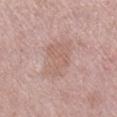Case summary:
- biopsy status: no biopsy performed (imaged during a skin exam)
- size: about 5 mm
- image: ~15 mm tile from a whole-body skin photo
- TBP lesion metrics: border irregularity of about 5 on a 0–10 scale, a color-variation rating of about 2/10, and peripheral color asymmetry of about 0.5; a lesion-detection confidence of about 100/100
- subject: female, aged around 50
- tile lighting: white-light
- site: the right lower leg